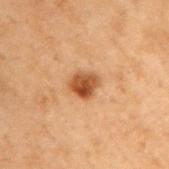Recorded during total-body skin imaging; not selected for excision or biopsy. A 15 mm close-up extracted from a 3D total-body photography capture. On the right upper arm. The subject is a male aged 68–72.